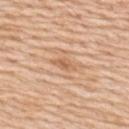notes: total-body-photography surveillance lesion; no biopsy | lighting: white-light illumination | TBP lesion metrics: a footprint of about 3 mm² and a symmetry-axis asymmetry near 0.25; an average lesion color of about L≈62 a*≈21 b*≈36 (CIELAB), a lesion–skin lightness drop of about 9, and a normalized border contrast of about 6; border irregularity of about 3 on a 0–10 scale, a within-lesion color-variation index near 1.5/10, and radial color variation of about 0.5 | subject: male, aged 58 to 62 | lesion diameter: ~3 mm (longest diameter) | image: ~15 mm crop, total-body skin-cancer survey | site: the back.